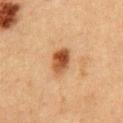Assessment:
Part of a total-body skin-imaging series; this lesion was reviewed on a skin check and was not flagged for biopsy.
Context:
This image is a 15 mm lesion crop taken from a total-body photograph. The patient is a male aged approximately 50. Located on the front of the torso.